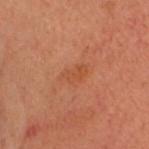Imaged during a routine full-body skin examination; the lesion was not biopsied and no histopathology is available.
A lesion tile, about 15 mm wide, cut from a 3D total-body photograph.
A female patient, roughly 50 years of age.
The lesion is on the head or neck.